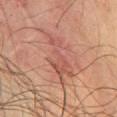• subject: male, aged approximately 70
• lesion diameter: about 7 mm
• location: the front of the torso
• lighting: cross-polarized illumination
• image: ~15 mm tile from a whole-body skin photo